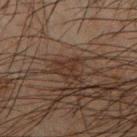Q: Was this lesion biopsied?
A: imaged on a skin check; not biopsied
Q: Illumination type?
A: cross-polarized
Q: How was this image acquired?
A: ~15 mm crop, total-body skin-cancer survey
Q: Where on the body is the lesion?
A: the right forearm
Q: What are the patient's age and sex?
A: male, aged 63 to 67
Q: Lesion size?
A: ≈2.5 mm